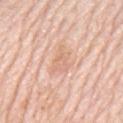biopsy status: imaged on a skin check; not biopsied | automated metrics: an area of roughly 5.5 mm² and an eccentricity of roughly 0.85 | body site: the mid back | image source: ~15 mm tile from a whole-body skin photo | lesion diameter: ~4 mm (longest diameter) | tile lighting: white-light | patient: male, aged approximately 80.